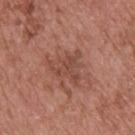notes — no biopsy performed (imaged during a skin exam); TBP lesion metrics — a footprint of about 8 mm², an outline eccentricity of about 0.35 (0 = round, 1 = elongated), and a shape-asymmetry score of about 0.55 (0 = symmetric); site — the upper back; patient — male, about 70 years old; illumination — white-light; image — ~15 mm crop, total-body skin-cancer survey; lesion size — ~4 mm (longest diameter).